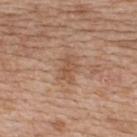Assessment:
Imaged during a routine full-body skin examination; the lesion was not biopsied and no histopathology is available.
Clinical summary:
A lesion tile, about 15 mm wide, cut from a 3D total-body photograph. The lesion is located on the upper back. Measured at roughly 3.5 mm in maximum diameter. A female subject, aged approximately 40.